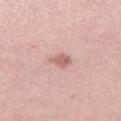Assessment:
Recorded during total-body skin imaging; not selected for excision or biopsy.
Clinical summary:
Measured at roughly 3 mm in maximum diameter. The lesion is located on the left thigh. Imaged with white-light lighting. A close-up tile cropped from a whole-body skin photograph, about 15 mm across. The subject is a female in their mid-40s.